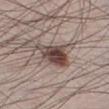Impression: Captured during whole-body skin photography for melanoma surveillance; the lesion was not biopsied. Image and clinical context: Located on the leg. The total-body-photography lesion software estimated border irregularity of about 4.5 on a 0–10 scale, a color-variation rating of about 7.5/10, and radial color variation of about 2.5. The analysis additionally found a classifier nevus-likeness of about 95/100 and a detector confidence of about 100 out of 100 that the crop contains a lesion. Captured under white-light illumination. Cropped from a total-body skin-imaging series; the visible field is about 15 mm. A male patient aged around 35. Measured at roughly 5 mm in maximum diameter.